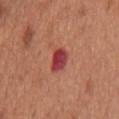Q: Was a biopsy performed?
A: catalogued during a skin exam; not biopsied
Q: Who is the patient?
A: male, aged 63–67
Q: Automated lesion metrics?
A: a border-irregularity rating of about 2/10 and a peripheral color-asymmetry measure near 1; an automated nevus-likeness rating near 0 out of 100 and lesion-presence confidence of about 100/100
Q: Illumination type?
A: white-light
Q: How large is the lesion?
A: about 3 mm
Q: What is the imaging modality?
A: ~15 mm tile from a whole-body skin photo
Q: What is the anatomic site?
A: the mid back